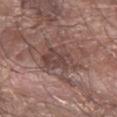<lesion>
  <biopsy_status>not biopsied; imaged during a skin examination</biopsy_status>
  <site>right forearm</site>
  <automated_metrics>
    <area_mm2_approx>12.0</area_mm2_approx>
    <eccentricity>0.65</eccentricity>
    <shape_asymmetry>0.35</shape_asymmetry>
    <cielab_L>44</cielab_L>
    <cielab_a>18</cielab_a>
    <cielab_b>21</cielab_b>
    <vs_skin_darker_L>8.0</vs_skin_darker_L>
    <vs_skin_contrast_norm>6.5</vs_skin_contrast_norm>
    <border_irregularity_0_10>4.5</border_irregularity_0_10>
    <color_variation_0_10>4.0</color_variation_0_10>
    <peripheral_color_asymmetry>1.5</peripheral_color_asymmetry>
    <nevus_likeness_0_100>0</nevus_likeness_0_100>
    <lesion_detection_confidence_0_100>90</lesion_detection_confidence_0_100>
  </automated_metrics>
  <image>
    <source>total-body photography crop</source>
    <field_of_view_mm>15</field_of_view_mm>
  </image>
  <lesion_size>
    <long_diameter_mm_approx>5.0</long_diameter_mm_approx>
  </lesion_size>
  <patient>
    <sex>male</sex>
    <age_approx>70</age_approx>
  </patient>
</lesion>